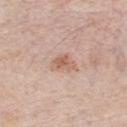workup: catalogued during a skin exam; not biopsied.